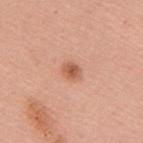Impression:
Recorded during total-body skin imaging; not selected for excision or biopsy.
Image and clinical context:
A female subject, in their 60s. A 15 mm crop from a total-body photograph taken for skin-cancer surveillance. Automated image analysis of the tile measured a footprint of about 3.5 mm², an outline eccentricity of about 0.65 (0 = round, 1 = elongated), and a shape-asymmetry score of about 0.3 (0 = symmetric). The software also gave a lesion color around L≈58 a*≈26 b*≈33 in CIELAB and a normalized border contrast of about 7.5. The analysis additionally found border irregularity of about 2 on a 0–10 scale, a within-lesion color-variation index near 3/10, and peripheral color asymmetry of about 1. The software also gave a nevus-likeness score of about 95/100 and a detector confidence of about 100 out of 100 that the crop contains a lesion. Imaged with white-light lighting. On the mid back.